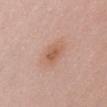Clinical impression: Captured during whole-body skin photography for melanoma surveillance; the lesion was not biopsied. Acquisition and patient details: The lesion-visualizer software estimated a lesion area of about 4.5 mm², an outline eccentricity of about 0.8 (0 = round, 1 = elongated), and a symmetry-axis asymmetry near 0.25. The analysis additionally found a lesion color around L≈58 a*≈23 b*≈30 in CIELAB, a lesion–skin lightness drop of about 9, and a normalized border contrast of about 6.5. It also reported a classifier nevus-likeness of about 80/100 and a detector confidence of about 100 out of 100 that the crop contains a lesion. A close-up tile cropped from a whole-body skin photograph, about 15 mm across. Located on the front of the torso. A female patient in their 40s.